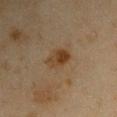{"biopsy_status": "not biopsied; imaged during a skin examination", "site": "arm", "automated_metrics": {"area_mm2_approx": 6.0, "eccentricity": 0.7, "shape_asymmetry": 0.15, "nevus_likeness_0_100": 85, "lesion_detection_confidence_0_100": 100}, "lesion_size": {"long_diameter_mm_approx": 3.5}, "lighting": "cross-polarized", "patient": {"sex": "male", "age_approx": 45}, "image": {"source": "total-body photography crop", "field_of_view_mm": 15}}